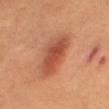This lesion was catalogued during total-body skin photography and was not selected for biopsy. The patient is a female approximately 40 years of age. A close-up tile cropped from a whole-body skin photograph, about 15 mm across. The tile uses cross-polarized illumination. The lesion is on the leg. Automated image analysis of the tile measured a footprint of about 15 mm², a shape eccentricity near 0.9, and two-axis asymmetry of about 0.2. It also reported a lesion color around L≈51 a*≈28 b*≈34 in CIELAB, a lesion–skin lightness drop of about 12, and a normalized border contrast of about 8. And it measured a nevus-likeness score of about 100/100 and a detector confidence of about 100 out of 100 that the crop contains a lesion. The lesion's longest dimension is about 7 mm.